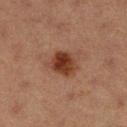Case summary:
- notes · imaged on a skin check; not biopsied
- body site · the right lower leg
- automated lesion analysis · a footprint of about 8.5 mm², an eccentricity of roughly 0.4, and two-axis asymmetry of about 0.2; a mean CIELAB color near L≈31 a*≈20 b*≈26, roughly 11 lightness units darker than nearby skin, and a normalized border contrast of about 10.5
- illumination · cross-polarized illumination
- diameter · ~3.5 mm (longest diameter)
- subject · female, aged 38–42
- acquisition · 15 mm crop, total-body photography